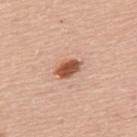workup=imaged on a skin check; not biopsied | patient=male, roughly 60 years of age | lesion diameter=~3 mm (longest diameter) | image=~15 mm crop, total-body skin-cancer survey | lighting=white-light | body site=the upper back.